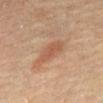Impression:
Recorded during total-body skin imaging; not selected for excision or biopsy.
Context:
A lesion tile, about 15 mm wide, cut from a 3D total-body photograph. This is a cross-polarized tile. The lesion is on the front of the torso. A male subject aged 58 to 62. Measured at roughly 4 mm in maximum diameter.